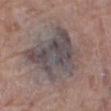{"biopsy_status": "not biopsied; imaged during a skin examination", "site": "right thigh", "lesion_size": {"long_diameter_mm_approx": 8.0}, "image": {"source": "total-body photography crop", "field_of_view_mm": 15}, "patient": {"sex": "female", "age_approx": 70}, "lighting": "white-light"}